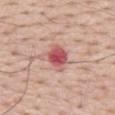  biopsy_status: not biopsied; imaged during a skin examination
  image:
    source: total-body photography crop
    field_of_view_mm: 15
  site: mid back
  automated_metrics:
    cielab_L: 55
    cielab_a: 32
    cielab_b: 24
    vs_skin_darker_L: 14.0
    vs_skin_contrast_norm: 9.0
    nevus_likeness_0_100: 0
    lesion_detection_confidence_0_100: 100
  lighting: white-light
  patient:
    sex: male
    age_approx: 65
  lesion_size:
    long_diameter_mm_approx: 3.0